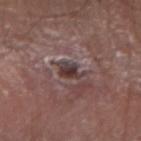Assessment: No biopsy was performed on this lesion — it was imaged during a full skin examination and was not determined to be concerning. Background: A male subject, aged 63–67. The lesion is located on the right forearm. This image is a 15 mm lesion crop taken from a total-body photograph.